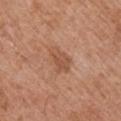notes: catalogued during a skin exam; not biopsied.